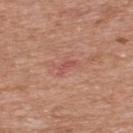Clinical impression:
Recorded during total-body skin imaging; not selected for excision or biopsy.
Context:
The patient is a male approximately 55 years of age. The tile uses white-light illumination. Longest diameter approximately 2.5 mm. Located on the upper back. This image is a 15 mm lesion crop taken from a total-body photograph.